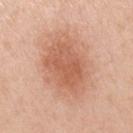workup: no biopsy performed (imaged during a skin exam)
patient: male, aged around 50
image: 15 mm crop, total-body photography
lesion diameter: about 8.5 mm
site: the right upper arm
illumination: white-light illumination